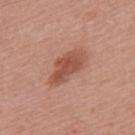From the upper back. Longest diameter approximately 5 mm. This is a white-light tile. An algorithmic analysis of the crop reported an area of roughly 10 mm², an eccentricity of roughly 0.9, and a shape-asymmetry score of about 0.3 (0 = symmetric). The analysis additionally found border irregularity of about 3.5 on a 0–10 scale and a color-variation rating of about 3/10. A male patient aged 48–52. Cropped from a whole-body photographic skin survey; the tile spans about 15 mm.